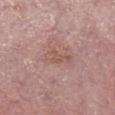The lesion was tiled from a total-body skin photograph and was not biopsied. The recorded lesion diameter is about 3.5 mm. The lesion is located on the right lower leg. A 15 mm crop from a total-body photograph taken for skin-cancer surveillance. A male patient, roughly 55 years of age.